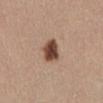No biopsy was performed on this lesion — it was imaged during a full skin examination and was not determined to be concerning. About 3 mm across. On the abdomen. A female patient, aged approximately 45. A close-up tile cropped from a whole-body skin photograph, about 15 mm across. Automated tile analysis of the lesion measured an area of roughly 6.5 mm² and an eccentricity of roughly 0.7. It also reported a lesion color around L≈45 a*≈20 b*≈27 in CIELAB, roughly 18 lightness units darker than nearby skin, and a normalized lesion–skin contrast near 12.5. And it measured a border-irregularity index near 2/10, internal color variation of about 3.5 on a 0–10 scale, and radial color variation of about 1. The analysis additionally found a classifier nevus-likeness of about 100/100.